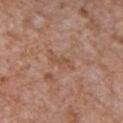The lesion was photographed on a routine skin check and not biopsied; there is no pathology result.
Located on the chest.
The patient is a male about 65 years old.
Imaged with white-light lighting.
A region of skin cropped from a whole-body photographic capture, roughly 15 mm wide.
Longest diameter approximately 3 mm.
Automated image analysis of the tile measured a footprint of about 2.5 mm² and a shape eccentricity near 0.95.